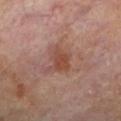{"image": {"source": "total-body photography crop", "field_of_view_mm": 15}, "lesion_size": {"long_diameter_mm_approx": 3.5}, "automated_metrics": {"area_mm2_approx": 6.5, "eccentricity": 0.7, "cielab_L": 45, "cielab_a": 22, "cielab_b": 27, "vs_skin_darker_L": 8.0, "vs_skin_contrast_norm": 7.0, "border_irregularity_0_10": 4.0, "color_variation_0_10": 2.5, "peripheral_color_asymmetry": 1.0}, "site": "left lower leg", "patient": {"sex": "male", "age_approx": 65}}